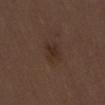Q: Was this lesion biopsied?
A: catalogued during a skin exam; not biopsied
Q: What are the patient's age and sex?
A: female, in their 50s
Q: Lesion location?
A: the abdomen
Q: What kind of image is this?
A: 15 mm crop, total-body photography